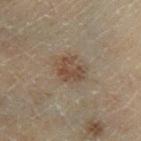notes: catalogued during a skin exam; not biopsied
patient: male, aged around 70
acquisition: ~15 mm crop, total-body skin-cancer survey
image-analysis metrics: a border-irregularity index near 2.5/10 and a peripheral color-asymmetry measure near 1; a nevus-likeness score of about 20/100
anatomic site: the left lower leg